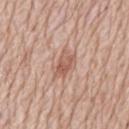Clinical impression:
Part of a total-body skin-imaging series; this lesion was reviewed on a skin check and was not flagged for biopsy.
Background:
Approximately 3.5 mm at its widest. A male patient, approximately 80 years of age. Automated tile analysis of the lesion measured an area of roughly 6 mm², an eccentricity of roughly 0.85, and two-axis asymmetry of about 0.3. And it measured roughly 10 lightness units darker than nearby skin and a normalized border contrast of about 6.5. The analysis additionally found border irregularity of about 3.5 on a 0–10 scale and radial color variation of about 1.5. It also reported an automated nevus-likeness rating near 35 out of 100. A 15 mm crop from a total-body photograph taken for skin-cancer surveillance. This is a white-light tile. Located on the back.